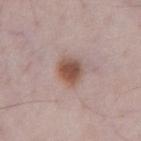Assessment:
Imaged during a routine full-body skin examination; the lesion was not biopsied and no histopathology is available.
Context:
This image is a 15 mm lesion crop taken from a total-body photograph. Captured under white-light illumination. About 3 mm across. The lesion is located on the abdomen. The total-body-photography lesion software estimated a border-irregularity rating of about 1.5/10, a within-lesion color-variation index near 4.5/10, and peripheral color asymmetry of about 1. The patient is a male roughly 70 years of age.